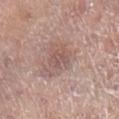Q: Was this lesion biopsied?
A: imaged on a skin check; not biopsied
Q: Where on the body is the lesion?
A: the right lower leg
Q: Lesion size?
A: ≈2.5 mm
Q: What is the imaging modality?
A: ~15 mm tile from a whole-body skin photo
Q: Patient demographics?
A: female, aged 83 or older